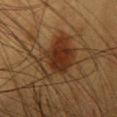Assessment: No biopsy was performed on this lesion — it was imaged during a full skin examination and was not determined to be concerning. Context: Automated tile analysis of the lesion measured an average lesion color of about L≈27 a*≈17 b*≈27 (CIELAB) and a normalized lesion–skin contrast near 9. And it measured a nevus-likeness score of about 95/100 and a detector confidence of about 100 out of 100 that the crop contains a lesion. Captured under cross-polarized illumination. A female subject aged around 40. Measured at roughly 6.5 mm in maximum diameter. Located on the head or neck. Cropped from a whole-body photographic skin survey; the tile spans about 15 mm.